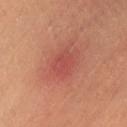Clinical summary:
A region of skin cropped from a whole-body photographic capture, roughly 15 mm wide. A female patient, aged 28–32. The lesion is on the leg.